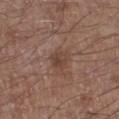- biopsy status · imaged on a skin check; not biopsied
- imaging modality · total-body-photography crop, ~15 mm field of view
- subject · male, about 55 years old
- image-analysis metrics · a footprint of about 4.5 mm² and an eccentricity of roughly 0.55; a color-variation rating of about 4/10 and radial color variation of about 1.5; a nevus-likeness score of about 0/100 and a lesion-detection confidence of about 100/100
- size · about 2.5 mm
- illumination · white-light illumination
- location · the left lower leg